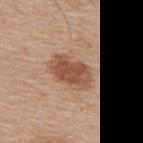Impression:
No biopsy was performed on this lesion — it was imaged during a full skin examination and was not determined to be concerning.
Image and clinical context:
A male subject, aged around 65. About 5 mm across. Automated tile analysis of the lesion measured an area of roughly 11 mm², an outline eccentricity of about 0.85 (0 = round, 1 = elongated), and a symmetry-axis asymmetry near 0.2. The analysis additionally found a lesion color around L≈51 a*≈21 b*≈32 in CIELAB, a lesion–skin lightness drop of about 13, and a lesion-to-skin contrast of about 9 (normalized; higher = more distinct). The analysis additionally found border irregularity of about 2.5 on a 0–10 scale, internal color variation of about 3 on a 0–10 scale, and a peripheral color-asymmetry measure near 1. The analysis additionally found a nevus-likeness score of about 80/100 and a detector confidence of about 100 out of 100 that the crop contains a lesion. This image is a 15 mm lesion crop taken from a total-body photograph. The lesion is located on the back. Captured under white-light illumination.